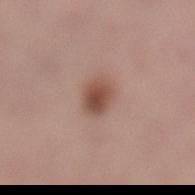{"lesion_size": {"long_diameter_mm_approx": 2.5}, "image": {"source": "total-body photography crop", "field_of_view_mm": 15}, "site": "leg", "patient": {"sex": "female", "age_approx": 35}}